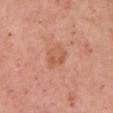Imaged during a routine full-body skin examination; the lesion was not biopsied and no histopathology is available. Measured at roughly 3.5 mm in maximum diameter. From the right upper arm. The patient is a female roughly 65 years of age. A 15 mm crop from a total-body photograph taken for skin-cancer surveillance. Imaged with white-light lighting.